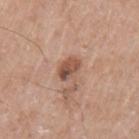Assessment: Captured during whole-body skin photography for melanoma surveillance; the lesion was not biopsied. Acquisition and patient details: A male subject, in their mid-60s. A region of skin cropped from a whole-body photographic capture, roughly 15 mm wide. Longest diameter approximately 3 mm. From the left upper arm. Captured under white-light illumination.